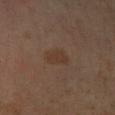This lesion was catalogued during total-body skin photography and was not selected for biopsy. The lesion is located on the left lower leg. A male patient, roughly 60 years of age. Captured under cross-polarized illumination. The recorded lesion diameter is about 3 mm. A 15 mm crop from a total-body photograph taken for skin-cancer surveillance.